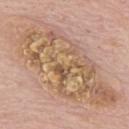No biopsy was performed on this lesion — it was imaged during a full skin examination and was not determined to be concerning. The tile uses white-light illumination. The recorded lesion diameter is about 15 mm. A male subject, approximately 75 years of age. Cropped from a whole-body photographic skin survey; the tile spans about 15 mm. From the upper back.